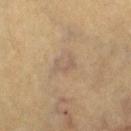The lesion was photographed on a routine skin check and not biopsied; there is no pathology result. The lesion's longest dimension is about 3 mm. A close-up tile cropped from a whole-body skin photograph, about 15 mm across. Imaged with cross-polarized lighting. A female subject, approximately 55 years of age. On the right thigh.